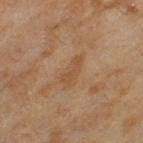<tbp_lesion>
  <image>
    <source>total-body photography crop</source>
    <field_of_view_mm>15</field_of_view_mm>
  </image>
  <site>leg</site>
  <patient>
    <sex>female</sex>
    <age_approx>60</age_approx>
  </patient>
  <lesion_size>
    <long_diameter_mm_approx>3.5</long_diameter_mm_approx>
  </lesion_size>
</tbp_lesion>